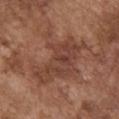Findings:
• workup · no biopsy performed (imaged during a skin exam)
• acquisition · total-body-photography crop, ~15 mm field of view
• lesion diameter · about 7.5 mm
• site · the chest
• patient · male, in their mid-70s
• tile lighting · white-light illumination